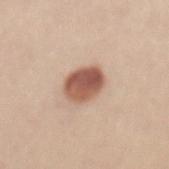{"biopsy_status": "not biopsied; imaged during a skin examination", "automated_metrics": {"area_mm2_approx": 11.0, "shape_asymmetry": 0.15, "nevus_likeness_0_100": 100}, "patient": {"sex": "female", "age_approx": 25}, "lesion_size": {"long_diameter_mm_approx": 4.0}, "lighting": "white-light", "site": "mid back", "image": {"source": "total-body photography crop", "field_of_view_mm": 15}}